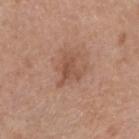A male patient, in their mid- to late 50s. On the arm. A 15 mm crop from a total-body photograph taken for skin-cancer surveillance. Measured at roughly 3.5 mm in maximum diameter.